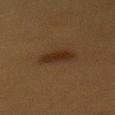biopsy_status: not biopsied; imaged during a skin examination
site: mid back
patient:
  sex: female
  age_approx: 40
image:
  source: total-body photography crop
  field_of_view_mm: 15